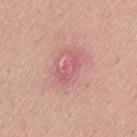Impression:
The lesion was tiled from a total-body skin photograph and was not biopsied.
Acquisition and patient details:
Captured under white-light illumination. A close-up tile cropped from a whole-body skin photograph, about 15 mm across. The lesion is on the lower back. Measured at roughly 4 mm in maximum diameter. A female patient, aged around 30. The lesion-visualizer software estimated a footprint of about 6 mm², an eccentricity of roughly 0.85, and a shape-asymmetry score of about 0.4 (0 = symmetric). The software also gave a border-irregularity rating of about 5.5/10 and internal color variation of about 2 on a 0–10 scale.